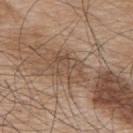Impression: The lesion was photographed on a routine skin check and not biopsied; there is no pathology result. Context: The lesion's longest dimension is about 4.5 mm. Cropped from a total-body skin-imaging series; the visible field is about 15 mm. A male patient roughly 75 years of age. The lesion is located on the upper back. The lesion-visualizer software estimated an area of roughly 9 mm², an eccentricity of roughly 0.8, and two-axis asymmetry of about 0.3. The software also gave a lesion color around L≈49 a*≈16 b*≈28 in CIELAB and roughly 7 lightness units darker than nearby skin. And it measured a border-irregularity rating of about 4.5/10 and a color-variation rating of about 3.5/10. Captured under white-light illumination.